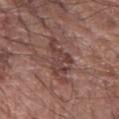Notes:
* workup — catalogued during a skin exam; not biopsied
* body site — the right forearm
* size — ≈5 mm
* imaging modality — 15 mm crop, total-body photography
* subject — male, roughly 70 years of age